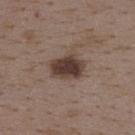notes — no biopsy performed (imaged during a skin exam) | patient — female, aged 33–37 | body site — the upper back | acquisition — ~15 mm crop, total-body skin-cancer survey | automated metrics — a footprint of about 10 mm² and a shape-asymmetry score of about 0.15 (0 = symmetric) | size — about 4 mm.